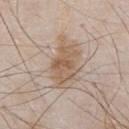follow-up = total-body-photography surveillance lesion; no biopsy
imaging modality = ~15 mm tile from a whole-body skin photo
diameter = ~5.5 mm (longest diameter)
anatomic site = the chest
subject = male, in their mid-50s